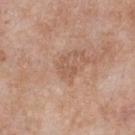Impression: Imaged during a routine full-body skin examination; the lesion was not biopsied and no histopathology is available. Image and clinical context: Measured at roughly 2.5 mm in maximum diameter. Automated image analysis of the tile measured a footprint of about 3 mm², an eccentricity of roughly 0.75, and two-axis asymmetry of about 0.55. The analysis additionally found a mean CIELAB color near L≈56 a*≈20 b*≈31 and a normalized border contrast of about 5. It also reported a border-irregularity rating of about 5/10, internal color variation of about 0 on a 0–10 scale, and radial color variation of about 0. The patient is a male in their 50s. The tile uses white-light illumination. The lesion is located on the chest. A 15 mm close-up extracted from a 3D total-body photography capture.